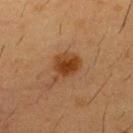{
  "biopsy_status": "not biopsied; imaged during a skin examination",
  "automated_metrics": {
    "shape_asymmetry": 0.3,
    "vs_skin_darker_L": 9.0,
    "vs_skin_contrast_norm": 9.0,
    "peripheral_color_asymmetry": 1.0,
    "nevus_likeness_0_100": 95,
    "lesion_detection_confidence_0_100": 100
  },
  "lighting": "cross-polarized",
  "site": "chest",
  "image": {
    "source": "total-body photography crop",
    "field_of_view_mm": 15
  },
  "patient": {
    "sex": "male",
    "age_approx": 55
  },
  "lesion_size": {
    "long_diameter_mm_approx": 4.0
  }
}